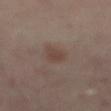Case summary:
• workup: catalogued during a skin exam; not biopsied
• image-analysis metrics: a shape eccentricity near 0.85 and a shape-asymmetry score of about 0.35 (0 = symmetric); a nevus-likeness score of about 55/100 and lesion-presence confidence of about 100/100
• size: ~3.5 mm (longest diameter)
• patient: aged approximately 55
• site: the mid back
• imaging modality: 15 mm crop, total-body photography